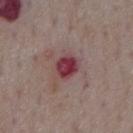<record>
<biopsy_status>not biopsied; imaged during a skin examination</biopsy_status>
<lighting>white-light</lighting>
<automated_metrics>
  <area_mm2_approx>7.0</area_mm2_approx>
  <shape_asymmetry>0.15</shape_asymmetry>
  <cielab_L>39</cielab_L>
  <cielab_a>28</cielab_a>
  <cielab_b>16</cielab_b>
  <vs_skin_darker_L>13.0</vs_skin_darker_L>
  <vs_skin_contrast_norm>10.5</vs_skin_contrast_norm>
  <border_irregularity_0_10>1.5</border_irregularity_0_10>
  <nevus_likeness_0_100>0</nevus_likeness_0_100>
</automated_metrics>
<site>chest</site>
<lesion_size>
  <long_diameter_mm_approx>3.5</long_diameter_mm_approx>
</lesion_size>
<image>
  <source>total-body photography crop</source>
  <field_of_view_mm>15</field_of_view_mm>
</image>
<patient>
  <sex>male</sex>
  <age_approx>75</age_approx>
</patient>
</record>